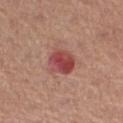image-analysis metrics: an area of roughly 8.5 mm², a shape eccentricity near 0.55, and a shape-asymmetry score of about 0.15 (0 = symmetric); a border-irregularity rating of about 1.5/10, internal color variation of about 5.5 on a 0–10 scale, and a peripheral color-asymmetry measure near 1.5; an automated nevus-likeness rating near 0 out of 100 and a lesion-detection confidence of about 100/100
image source: total-body-photography crop, ~15 mm field of view
diameter: about 3.5 mm
tile lighting: white-light
location: the right thigh
subject: female, approximately 65 years of age